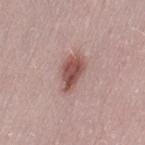Impression: This lesion was catalogued during total-body skin photography and was not selected for biopsy. Context: Automated tile analysis of the lesion measured a lesion color around L≈52 a*≈22 b*≈23 in CIELAB and a normalized border contrast of about 9.5. It also reported a border-irregularity rating of about 2.5/10, internal color variation of about 4 on a 0–10 scale, and radial color variation of about 1.5. Captured under white-light illumination. About 4.5 mm across. A 15 mm close-up extracted from a 3D total-body photography capture. The subject is a female aged around 30. Located on the leg.